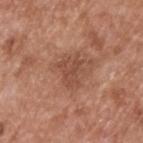biopsy_status: not biopsied; imaged during a skin examination
image:
  source: total-body photography crop
  field_of_view_mm: 15
patient:
  sex: male
  age_approx: 65
site: back